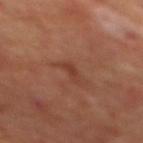  biopsy_status: not biopsied; imaged during a skin examination
  site: mid back
  patient:
    sex: male
    age_approx: 70
  lighting: cross-polarized
  automated_metrics:
    eccentricity: 0.95
    nevus_likeness_0_100: 0
    lesion_detection_confidence_0_100: 100
  image:
    source: total-body photography crop
    field_of_view_mm: 15
  lesion_size:
    long_diameter_mm_approx: 2.5